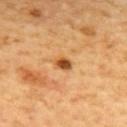notes: no biopsy performed (imaged during a skin exam)
automated lesion analysis: a lesion area of about 3 mm², an eccentricity of roughly 0.6, and a shape-asymmetry score of about 0.25 (0 = symmetric); a lesion color around L≈52 a*≈25 b*≈43 in CIELAB, roughly 15 lightness units darker than nearby skin, and a lesion-to-skin contrast of about 10 (normalized; higher = more distinct); a border-irregularity index near 2/10, a within-lesion color-variation index near 4/10, and radial color variation of about 1.5; an automated nevus-likeness rating near 90 out of 100 and a lesion-detection confidence of about 100/100
image: ~15 mm crop, total-body skin-cancer survey
tile lighting: cross-polarized illumination
body site: the mid back
diameter: about 2 mm
patient: male, aged 58 to 62